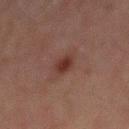Q: Was this lesion biopsied?
A: no biopsy performed (imaged during a skin exam)
Q: What is the anatomic site?
A: the mid back
Q: Patient demographics?
A: male, about 60 years old
Q: How was this image acquired?
A: ~15 mm tile from a whole-body skin photo